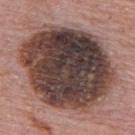notes — total-body-photography surveillance lesion; no biopsy
body site — the upper back
subject — male, aged around 75
image source — total-body-photography crop, ~15 mm field of view